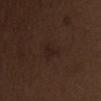Assessment: Part of a total-body skin-imaging series; this lesion was reviewed on a skin check and was not flagged for biopsy. Image and clinical context: A roughly 15 mm field-of-view crop from a total-body skin photograph. The patient is a male aged approximately 70. Imaged with white-light lighting. The recorded lesion diameter is about 3 mm. On the abdomen.